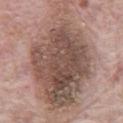follow-up=imaged on a skin check; not biopsied
patient=male, aged approximately 70
illumination=white-light
lesion diameter=~14 mm (longest diameter)
anatomic site=the front of the torso
imaging modality=total-body-photography crop, ~15 mm field of view